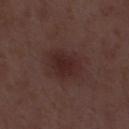Notes:
– biopsy status: catalogued during a skin exam; not biopsied
– image-analysis metrics: an area of roughly 10 mm², a shape eccentricity near 0.6, and a symmetry-axis asymmetry near 0.25; a lesion color around L≈25 a*≈19 b*≈18 in CIELAB, about 7 CIELAB-L* units darker than the surrounding skin, and a normalized lesion–skin contrast near 7.5; a border-irregularity rating of about 2.5/10 and a peripheral color-asymmetry measure near 0.5; a nevus-likeness score of about 20/100 and lesion-presence confidence of about 100/100
– tile lighting: white-light
– subject: male, aged 48–52
– image source: 15 mm crop, total-body photography
– lesion diameter: ≈4 mm